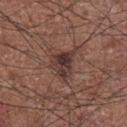Part of a total-body skin-imaging series; this lesion was reviewed on a skin check and was not flagged for biopsy.
A roughly 15 mm field-of-view crop from a total-body skin photograph.
The lesion is located on the chest.
The lesion-visualizer software estimated a border-irregularity index near 5/10, a within-lesion color-variation index near 5.5/10, and radial color variation of about 2.
Imaged with white-light lighting.
The subject is a male in their mid- to late 70s.